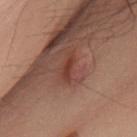The lesion was photographed on a routine skin check and not biopsied; there is no pathology result. Approximately 3 mm at its widest. A male subject, in their 50s. Cropped from a whole-body photographic skin survey; the tile spans about 15 mm. On the upper back. Imaged with white-light lighting.